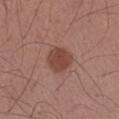Q: Is there a histopathology result?
A: imaged on a skin check; not biopsied
Q: What is the anatomic site?
A: the left forearm
Q: What are the patient's age and sex?
A: male, in their mid- to late 30s
Q: What is the imaging modality?
A: ~15 mm crop, total-body skin-cancer survey